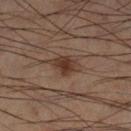No biopsy was performed on this lesion — it was imaged during a full skin examination and was not determined to be concerning.
From the left lower leg.
Approximately 3 mm at its widest.
A lesion tile, about 15 mm wide, cut from a 3D total-body photograph.
The tile uses cross-polarized illumination.
A male patient, aged approximately 60.